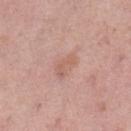Assessment:
The lesion was photographed on a routine skin check and not biopsied; there is no pathology result.
Clinical summary:
Cropped from a whole-body photographic skin survey; the tile spans about 15 mm. The lesion is on the left lower leg. The patient is a female aged approximately 50. Captured under white-light illumination.